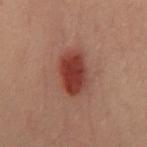| key | value |
|---|---|
| notes | total-body-photography surveillance lesion; no biopsy |
| anatomic site | the chest |
| image-analysis metrics | an average lesion color of about L≈31 a*≈23 b*≈22 (CIELAB) and a normalized lesion–skin contrast near 10.5; a border-irregularity rating of about 2/10 and a within-lesion color-variation index near 3.5/10 |
| diameter | ~5 mm (longest diameter) |
| imaging modality | ~15 mm tile from a whole-body skin photo |
| subject | male, roughly 40 years of age |